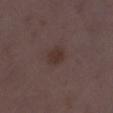follow-up: total-body-photography surveillance lesion; no biopsy
automated metrics: a mean CIELAB color near L≈32 a*≈15 b*≈19, about 6 CIELAB-L* units darker than the surrounding skin, and a normalized lesion–skin contrast near 7.5
acquisition: ~15 mm crop, total-body skin-cancer survey
anatomic site: the left thigh
subject: female, in their mid-30s
lighting: white-light